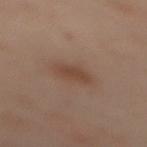Q: Was this lesion biopsied?
A: no biopsy performed (imaged during a skin exam)
Q: What is the anatomic site?
A: the back
Q: What is the imaging modality?
A: ~15 mm tile from a whole-body skin photo
Q: What are the patient's age and sex?
A: female, aged 53 to 57
Q: How large is the lesion?
A: ~3 mm (longest diameter)
Q: What did automated image analysis measure?
A: a shape eccentricity near 0.65 and a shape-asymmetry score of about 0.2 (0 = symmetric); a mean CIELAB color near L≈35 a*≈15 b*≈22 and roughly 6 lightness units darker than nearby skin